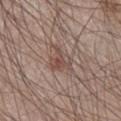notes — no biopsy performed (imaged during a skin exam)
image-analysis metrics — a footprint of about 6.5 mm² and two-axis asymmetry of about 0.4; a color-variation rating of about 5/10; an automated nevus-likeness rating near 5 out of 100
subject — male, in their 60s
imaging modality — ~15 mm crop, total-body skin-cancer survey
lesion diameter — about 3.5 mm
tile lighting — white-light illumination
site — the right lower leg